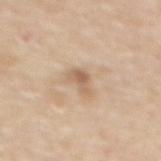Captured during whole-body skin photography for melanoma surveillance; the lesion was not biopsied.
A female subject in their mid- to late 60s.
Approximately 3 mm at its widest.
From the mid back.
The total-body-photography lesion software estimated a lesion area of about 3.5 mm², an eccentricity of roughly 0.85, and a shape-asymmetry score of about 0.4 (0 = symmetric). The software also gave a mean CIELAB color near L≈61 a*≈17 b*≈32 and a normalized lesion–skin contrast near 6.5. The software also gave a detector confidence of about 100 out of 100 that the crop contains a lesion.
Imaged with white-light lighting.
A region of skin cropped from a whole-body photographic capture, roughly 15 mm wide.